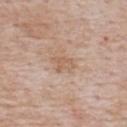Notes:
– notes — imaged on a skin check; not biopsied
– imaging modality — total-body-photography crop, ~15 mm field of view
– diameter — about 3 mm
– lighting — white-light illumination
– automated lesion analysis — a mean CIELAB color near L≈60 a*≈18 b*≈31, about 7 CIELAB-L* units darker than the surrounding skin, and a normalized border contrast of about 5.5; an automated nevus-likeness rating near 0 out of 100
– body site — the upper back
– subject — male, aged approximately 75